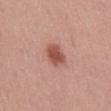Findings:
• body site: the mid back
• size: ~3 mm (longest diameter)
• image source: 15 mm crop, total-body photography
• subject: male, aged 53 to 57